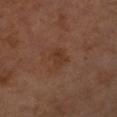The lesion was tiled from a total-body skin photograph and was not biopsied.
Located on the left forearm.
Longest diameter approximately 2.5 mm.
Automated tile analysis of the lesion measured a border-irregularity rating of about 3/10, internal color variation of about 2 on a 0–10 scale, and radial color variation of about 1. It also reported a classifier nevus-likeness of about 5/100 and lesion-presence confidence of about 100/100.
A female patient aged 53 to 57.
A close-up tile cropped from a whole-body skin photograph, about 15 mm across.